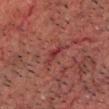Recorded during total-body skin imaging; not selected for excision or biopsy. A male patient in their 50s. Imaged with cross-polarized lighting. The lesion-visualizer software estimated an eccentricity of roughly 0.85 and two-axis asymmetry of about 0.4. It also reported a border-irregularity index near 4/10 and internal color variation of about 2.5 on a 0–10 scale. It also reported an automated nevus-likeness rating near 0 out of 100 and a lesion-detection confidence of about 55/100. On the head or neck. A close-up tile cropped from a whole-body skin photograph, about 15 mm across.